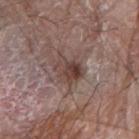workup = no biopsy performed (imaged during a skin exam) | TBP lesion metrics = an average lesion color of about L≈42 a*≈17 b*≈21 (CIELAB) and a lesion–skin lightness drop of about 10; a border-irregularity rating of about 3/10, a color-variation rating of about 7.5/10, and peripheral color asymmetry of about 3 | diameter = about 3.5 mm | site = the arm | patient = male, in their 80s | illumination = white-light | image source = total-body-photography crop, ~15 mm field of view.